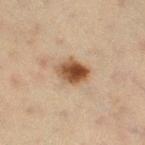biopsy_status: not biopsied; imaged during a skin examination
patient:
  sex: female
  age_approx: 40
lesion_size:
  long_diameter_mm_approx: 4.0
image:
  source: total-body photography crop
  field_of_view_mm: 15
site: leg
automated_metrics:
  cielab_L: 44
  cielab_a: 17
  cielab_b: 30
  vs_skin_darker_L: 14.0
  vs_skin_contrast_norm: 11.0
  peripheral_color_asymmetry: 3.0